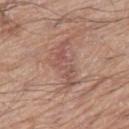Impression: Imaged during a routine full-body skin examination; the lesion was not biopsied and no histopathology is available. Clinical summary: On the leg. The patient is a male approximately 70 years of age. A lesion tile, about 15 mm wide, cut from a 3D total-body photograph.